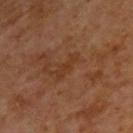• follow-up · catalogued during a skin exam; not biopsied
• patient · male, aged around 65
• lesion size · ≈3.5 mm
• site · the upper back
• lighting · cross-polarized
• TBP lesion metrics · a lesion color around L≈35 a*≈21 b*≈32 in CIELAB
• imaging modality · ~15 mm tile from a whole-body skin photo